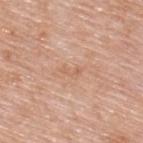size = ≈2.5 mm
patient = male, aged approximately 80
illumination = white-light illumination
body site = the upper back
image = ~15 mm tile from a whole-body skin photo